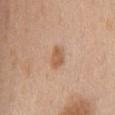Impression:
This lesion was catalogued during total-body skin photography and was not selected for biopsy.
Context:
From the chest. This image is a 15 mm lesion crop taken from a total-body photograph. The patient is a female aged around 65. Imaged with white-light lighting.